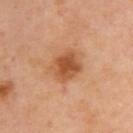Assessment:
Recorded during total-body skin imaging; not selected for excision or biopsy.
Image and clinical context:
The total-body-photography lesion software estimated an area of roughly 9 mm², a shape eccentricity near 0.45, and a shape-asymmetry score of about 0.15 (0 = symmetric). And it measured a lesion color around L≈54 a*≈26 b*≈39 in CIELAB and a lesion-to-skin contrast of about 8.5 (normalized; higher = more distinct). Longest diameter approximately 3.5 mm. This image is a 15 mm lesion crop taken from a total-body photograph. A female patient roughly 40 years of age. Captured under cross-polarized illumination. The lesion is located on the upper back.